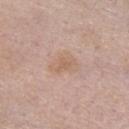Imaged during a routine full-body skin examination; the lesion was not biopsied and no histopathology is available. A female patient aged approximately 65. Longest diameter approximately 3.5 mm. A 15 mm crop from a total-body photograph taken for skin-cancer surveillance. An algorithmic analysis of the crop reported a footprint of about 7 mm² and an eccentricity of roughly 0.7. The software also gave a nevus-likeness score of about 0/100 and lesion-presence confidence of about 100/100. The lesion is on the chest.